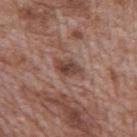Assessment: No biopsy was performed on this lesion — it was imaged during a full skin examination and was not determined to be concerning. Context: The lesion is located on the back. This image is a 15 mm lesion crop taken from a total-body photograph. Longest diameter approximately 3 mm. A male subject roughly 70 years of age. This is a white-light tile.